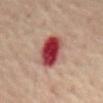- workup — catalogued during a skin exam; not biopsied